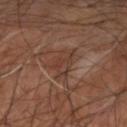Impression: No biopsy was performed on this lesion — it was imaged during a full skin examination and was not determined to be concerning. Image and clinical context: A 15 mm close-up extracted from a 3D total-body photography capture. The tile uses cross-polarized illumination. The patient is a male roughly 60 years of age. On the left arm.